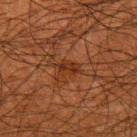Part of a total-body skin-imaging series; this lesion was reviewed on a skin check and was not flagged for biopsy. The lesion is located on the arm. A male patient, in their 50s. About 2.5 mm across. The tile uses cross-polarized illumination. A 15 mm close-up extracted from a 3D total-body photography capture.